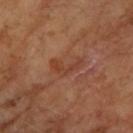Assessment: The lesion was tiled from a total-body skin photograph and was not biopsied. Acquisition and patient details: On the left forearm. A female subject aged approximately 70. An algorithmic analysis of the crop reported an average lesion color of about L≈43 a*≈24 b*≈32 (CIELAB), roughly 6 lightness units darker than nearby skin, and a normalized lesion–skin contrast near 5. And it measured a border-irregularity rating of about 6/10 and a color-variation rating of about 3/10. It also reported an automated nevus-likeness rating near 0 out of 100 and a lesion-detection confidence of about 100/100. Measured at roughly 4 mm in maximum diameter. A close-up tile cropped from a whole-body skin photograph, about 15 mm across.